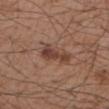This lesion was catalogued during total-body skin photography and was not selected for biopsy. From the right forearm. Imaged with white-light lighting. Longest diameter approximately 4 mm. Automated image analysis of the tile measured an area of roughly 6 mm² and two-axis asymmetry of about 0.45. The analysis additionally found about 10 CIELAB-L* units darker than the surrounding skin and a normalized border contrast of about 8.5. And it measured a border-irregularity rating of about 5/10 and a peripheral color-asymmetry measure near 0.5. The software also gave a nevus-likeness score of about 70/100 and lesion-presence confidence of about 100/100. The subject is a male aged approximately 55. A lesion tile, about 15 mm wide, cut from a 3D total-body photograph.